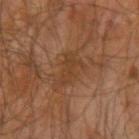Assessment: Part of a total-body skin-imaging series; this lesion was reviewed on a skin check and was not flagged for biopsy. Background: The lesion is on the right upper arm. Automated image analysis of the tile measured an area of roughly 5.5 mm², an outline eccentricity of about 0.85 (0 = round, 1 = elongated), and two-axis asymmetry of about 0.4. The analysis additionally found an average lesion color of about L≈37 a*≈19 b*≈30 (CIELAB), a lesion–skin lightness drop of about 6, and a normalized border contrast of about 5.5. The analysis additionally found a nevus-likeness score of about 0/100 and lesion-presence confidence of about 100/100. The patient is a male aged approximately 65. Cropped from a total-body skin-imaging series; the visible field is about 15 mm.